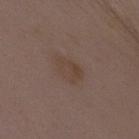  biopsy_status: not biopsied; imaged during a skin examination
  image:
    source: total-body photography crop
    field_of_view_mm: 15
  site: mid back
  lesion_size:
    long_diameter_mm_approx: 4.0
  patient:
    sex: female
    age_approx: 35
  lighting: white-light
  automated_metrics:
    area_mm2_approx: 7.0
    eccentricity: 0.85
    shape_asymmetry: 0.2
    border_irregularity_0_10: 2.0
    color_variation_0_10: 2.5
    peripheral_color_asymmetry: 1.0
    nevus_likeness_0_100: 5